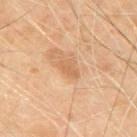The lesion was tiled from a total-body skin photograph and was not biopsied. A 15 mm crop from a total-body photograph taken for skin-cancer surveillance. The patient is a male aged approximately 70. The total-body-photography lesion software estimated a nevus-likeness score of about 0/100 and a detector confidence of about 100 out of 100 that the crop contains a lesion. The recorded lesion diameter is about 2.5 mm. Captured under cross-polarized illumination. The lesion is located on the upper back.